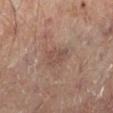Q: Is there a histopathology result?
A: catalogued during a skin exam; not biopsied
Q: Where on the body is the lesion?
A: the right lower leg
Q: How was this image acquired?
A: 15 mm crop, total-body photography
Q: Who is the patient?
A: male, aged 63–67
Q: What lighting was used for the tile?
A: cross-polarized illumination